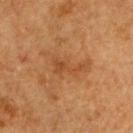follow-up = imaged on a skin check; not biopsied | lighting = cross-polarized illumination | automated metrics = an average lesion color of about L≈42 a*≈22 b*≈35 (CIELAB), roughly 6 lightness units darker than nearby skin, and a normalized lesion–skin contrast near 5.5 | image = ~15 mm crop, total-body skin-cancer survey | patient = male, in their mid- to late 80s or older | site = the head or neck.